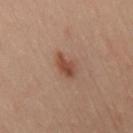| field | value |
|---|---|
| workup | total-body-photography surveillance lesion; no biopsy |
| image source | 15 mm crop, total-body photography |
| TBP lesion metrics | an area of roughly 4.5 mm², an outline eccentricity of about 0.9 (0 = round, 1 = elongated), and a symmetry-axis asymmetry near 0.3; a lesion–skin lightness drop of about 11 and a lesion-to-skin contrast of about 8.5 (normalized; higher = more distinct); a border-irregularity index near 3.5/10 and a peripheral color-asymmetry measure near 1 |
| subject | female, about 40 years old |
| lighting | cross-polarized illumination |
| location | the left leg |
| diameter | ≈3.5 mm |